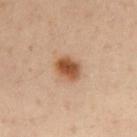A female subject aged approximately 55. Located on the chest. Captured under cross-polarized illumination. A lesion tile, about 15 mm wide, cut from a 3D total-body photograph. The recorded lesion diameter is about 3 mm.